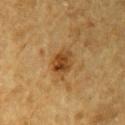workup: no biopsy performed (imaged during a skin exam)
illumination: cross-polarized
imaging modality: total-body-photography crop, ~15 mm field of view
subject: male, in their mid-80s or older
TBP lesion metrics: a footprint of about 7.5 mm², an outline eccentricity of about 0.75 (0 = round, 1 = elongated), and two-axis asymmetry of about 0.25; a mean CIELAB color near L≈39 a*≈18 b*≈36, about 10 CIELAB-L* units darker than the surrounding skin, and a lesion-to-skin contrast of about 8.5 (normalized; higher = more distinct); a border-irregularity rating of about 2.5/10, a color-variation rating of about 5.5/10, and a peripheral color-asymmetry measure near 2; an automated nevus-likeness rating near 75 out of 100 and a detector confidence of about 100 out of 100 that the crop contains a lesion
location: the right upper arm
lesion diameter: ≈4 mm